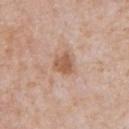follow-up: imaged on a skin check; not biopsied
diameter: ~2.5 mm (longest diameter)
anatomic site: the chest
illumination: white-light
acquisition: ~15 mm tile from a whole-body skin photo
patient: male, approximately 65 years of age